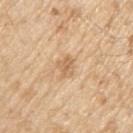<record>
  <biopsy_status>not biopsied; imaged during a skin examination</biopsy_status>
  <lesion_size>
    <long_diameter_mm_approx>2.5</long_diameter_mm_approx>
  </lesion_size>
  <site>right upper arm</site>
  <image>
    <source>total-body photography crop</source>
    <field_of_view_mm>15</field_of_view_mm>
  </image>
  <patient>
    <sex>male</sex>
    <age_approx>70</age_approx>
  </patient>
  <lighting>white-light</lighting>
  <automated_metrics>
    <border_irregularity_0_10>2.5</border_irregularity_0_10>
    <nevus_likeness_0_100>5</nevus_likeness_0_100>
    <lesion_detection_confidence_0_100>100</lesion_detection_confidence_0_100>
  </automated_metrics>
</record>